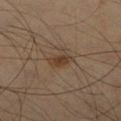Clinical impression: No biopsy was performed on this lesion — it was imaged during a full skin examination and was not determined to be concerning. Clinical summary: A 15 mm close-up extracted from a 3D total-body photography capture. An algorithmic analysis of the crop reported a footprint of about 4 mm², an outline eccentricity of about 0.8 (0 = round, 1 = elongated), and a symmetry-axis asymmetry near 0.25. The lesion is on the left lower leg. The tile uses cross-polarized illumination. A male patient, aged 58 to 62. The lesion's longest dimension is about 3 mm.